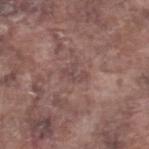- notes · catalogued during a skin exam; not biopsied
- lighting · white-light illumination
- size · about 2.5 mm
- anatomic site · the right lower leg
- TBP lesion metrics · an eccentricity of roughly 0.8; a mean CIELAB color near L≈46 a*≈18 b*≈19; a nevus-likeness score of about 0/100 and a detector confidence of about 65 out of 100 that the crop contains a lesion
- patient · male, about 75 years old
- image source · ~15 mm tile from a whole-body skin photo